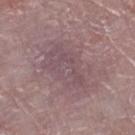The lesion was tiled from a total-body skin photograph and was not biopsied.
A female subject, roughly 65 years of age.
The lesion is located on the right lower leg.
Approximately 8 mm at its widest.
A 15 mm close-up extracted from a 3D total-body photography capture.